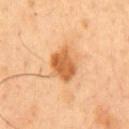| field | value |
|---|---|
| image-analysis metrics | a mean CIELAB color near L≈63 a*≈26 b*≈44, roughly 13 lightness units darker than nearby skin, and a normalized border contrast of about 8.5; a border-irregularity rating of about 1.5/10, a color-variation rating of about 5/10, and a peripheral color-asymmetry measure near 1.5 |
| patient | male, in their 50s |
| body site | the chest |
| lighting | cross-polarized illumination |
| image source | total-body-photography crop, ~15 mm field of view |